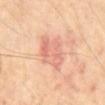The lesion was photographed on a routine skin check and not biopsied; there is no pathology result. The total-body-photography lesion software estimated a shape-asymmetry score of about 0.25 (0 = symmetric). The analysis additionally found a classifier nevus-likeness of about 0/100 and a detector confidence of about 100 out of 100 that the crop contains a lesion. A 15 mm crop from a total-body photograph taken for skin-cancer surveillance. The lesion is on the back. The tile uses cross-polarized illumination. Measured at roughly 6 mm in maximum diameter. A male patient, roughly 65 years of age.